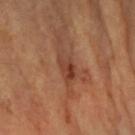Case summary:
– imaging modality: 15 mm crop, total-body photography
– subject: female, aged 58–62
– anatomic site: the left forearm